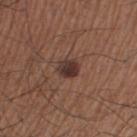{
  "biopsy_status": "not biopsied; imaged during a skin examination",
  "site": "leg",
  "patient": {
    "sex": "male",
    "age_approx": 65
  },
  "image": {
    "source": "total-body photography crop",
    "field_of_view_mm": 15
  }
}